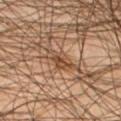Located on the left thigh.
A 15 mm crop from a total-body photograph taken for skin-cancer surveillance.
The patient is a male approximately 45 years of age.
Automated tile analysis of the lesion measured a shape eccentricity near 0.85. It also reported an average lesion color of about L≈47 a*≈19 b*≈31 (CIELAB), a lesion–skin lightness drop of about 9, and a normalized border contrast of about 7. And it measured a border-irregularity index near 3.5/10, a color-variation rating of about 4/10, and peripheral color asymmetry of about 1.5.